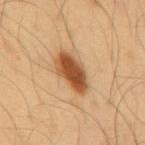follow-up: imaged on a skin check; not biopsied
subject: male, roughly 55 years of age
diameter: about 5 mm
automated lesion analysis: an average lesion color of about L≈50 a*≈23 b*≈37 (CIELAB), a lesion–skin lightness drop of about 17, and a lesion-to-skin contrast of about 11.5 (normalized; higher = more distinct)
imaging modality: ~15 mm crop, total-body skin-cancer survey
site: the mid back